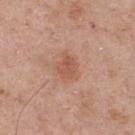The lesion was tiled from a total-body skin photograph and was not biopsied. A close-up tile cropped from a whole-body skin photograph, about 15 mm across. A male patient aged around 55. This is a white-light tile. The lesion is on the upper back. About 2.5 mm across.